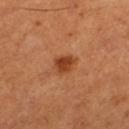  biopsy_status: not biopsied; imaged during a skin examination
  lesion_size:
    long_diameter_mm_approx: 3.0
  site: left thigh
  patient:
    sex: male
    age_approx: 50
  image:
    source: total-body photography crop
    field_of_view_mm: 15